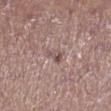Clinical summary:
The lesion is on the leg. Cropped from a whole-body photographic skin survey; the tile spans about 15 mm. An algorithmic analysis of the crop reported an area of roughly 3 mm², a shape eccentricity near 0.8, and a shape-asymmetry score of about 0.35 (0 = symmetric). And it measured a lesion color around L≈51 a*≈18 b*≈19 in CIELAB, roughly 9 lightness units darker than nearby skin, and a normalized border contrast of about 7. And it measured a nevus-likeness score of about 0/100. Captured under white-light illumination. Approximately 2.5 mm at its widest. The patient is a female roughly 20 years of age.